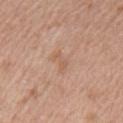The tile uses white-light illumination. About 2.5 mm across. The lesion is on the arm. The subject is a male in their 60s. A close-up tile cropped from a whole-body skin photograph, about 15 mm across.